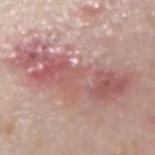follow-up: catalogued during a skin exam; not biopsied